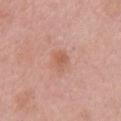Findings:
• notes: imaged on a skin check; not biopsied
• patient: female, approximately 70 years of age
• size: ~2.5 mm (longest diameter)
• location: the chest
• image source: 15 mm crop, total-body photography
• illumination: white-light